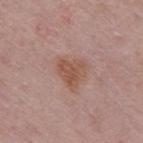Q: Was this lesion biopsied?
A: total-body-photography surveillance lesion; no biopsy
Q: Automated lesion metrics?
A: an eccentricity of roughly 0.4 and a symmetry-axis asymmetry near 0.45; an average lesion color of about L≈52 a*≈21 b*≈27 (CIELAB), roughly 8 lightness units darker than nearby skin, and a lesion-to-skin contrast of about 7 (normalized; higher = more distinct); a nevus-likeness score of about 15/100
Q: How was this image acquired?
A: ~15 mm crop, total-body skin-cancer survey
Q: Who is the patient?
A: female, aged 48–52
Q: What lighting was used for the tile?
A: white-light illumination
Q: Lesion size?
A: ≈3.5 mm
Q: Lesion location?
A: the upper back